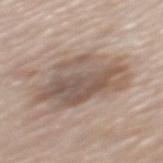Case summary:
• follow-up: total-body-photography surveillance lesion; no biopsy
• subject: male, aged 73–77
• lesion size: about 9 mm
• body site: the mid back
• image source: total-body-photography crop, ~15 mm field of view